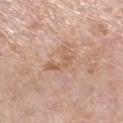Impression: No biopsy was performed on this lesion — it was imaged during a full skin examination and was not determined to be concerning. Clinical summary: The lesion's longest dimension is about 4 mm. From the left lower leg. An algorithmic analysis of the crop reported an average lesion color of about L≈60 a*≈20 b*≈31 (CIELAB), a lesion–skin lightness drop of about 8, and a normalized lesion–skin contrast near 6. The tile uses white-light illumination. A female subject in their 70s. A lesion tile, about 15 mm wide, cut from a 3D total-body photograph.